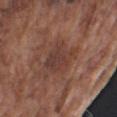This lesion was catalogued during total-body skin photography and was not selected for biopsy.
A region of skin cropped from a whole-body photographic capture, roughly 15 mm wide.
Imaged with white-light lighting.
A male subject aged 73–77.
From the right upper arm.